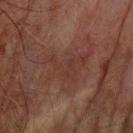The lesion was photographed on a routine skin check and not biopsied; there is no pathology result. This is a cross-polarized tile. Automated tile analysis of the lesion measured a within-lesion color-variation index near 1.5/10 and peripheral color asymmetry of about 0.5. The analysis additionally found an automated nevus-likeness rating near 0 out of 100 and a lesion-detection confidence of about 65/100. A male patient about 75 years old. Cropped from a total-body skin-imaging series; the visible field is about 15 mm. Measured at roughly 5.5 mm in maximum diameter. From the arm.